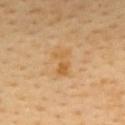Clinical impression: The lesion was tiled from a total-body skin photograph and was not biopsied. Context: A 15 mm close-up extracted from a 3D total-body photography capture. On the upper back. The subject is a female aged approximately 50.